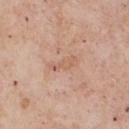This lesion was catalogued during total-body skin photography and was not selected for biopsy. A lesion tile, about 15 mm wide, cut from a 3D total-body photograph. This is a white-light tile. Automated tile analysis of the lesion measured an area of roughly 4 mm² and an eccentricity of roughly 0.95. About 3.5 mm across. A male patient, in their mid-50s. From the chest.